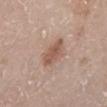Captured during whole-body skin photography for melanoma surveillance; the lesion was not biopsied. The total-body-photography lesion software estimated a border-irregularity index near 2/10, a within-lesion color-variation index near 3/10, and radial color variation of about 1. On the leg. A female subject in their mid- to late 60s. This is a white-light tile. Longest diameter approximately 4.5 mm. A roughly 15 mm field-of-view crop from a total-body skin photograph.